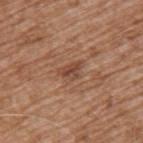Imaged with white-light lighting. Longest diameter approximately 2.5 mm. On the arm. A male subject, in their mid- to late 70s. This image is a 15 mm lesion crop taken from a total-body photograph. Automated tile analysis of the lesion measured a color-variation rating of about 3.5/10 and a peripheral color-asymmetry measure near 1. It also reported a classifier nevus-likeness of about 0/100 and a lesion-detection confidence of about 100/100.